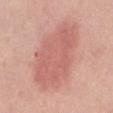Imaged during a routine full-body skin examination; the lesion was not biopsied and no histopathology is available. The recorded lesion diameter is about 10 mm. The total-body-photography lesion software estimated a symmetry-axis asymmetry near 0.2. It also reported an average lesion color of about L≈61 a*≈27 b*≈26 (CIELAB) and roughly 9 lightness units darker than nearby skin. It also reported a nevus-likeness score of about 90/100 and a detector confidence of about 100 out of 100 that the crop contains a lesion. The tile uses white-light illumination. The subject is a male aged 38–42. Cropped from a whole-body photographic skin survey; the tile spans about 15 mm. The lesion is on the mid back.